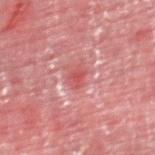| field | value |
|---|---|
| follow-up | imaged on a skin check; not biopsied |
| site | the leg |
| diameter | ~2.5 mm (longest diameter) |
| image | 15 mm crop, total-body photography |
| lighting | cross-polarized illumination |
| subject | male, about 55 years old |